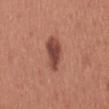Findings:
• body site: the abdomen
• size: ≈6 mm
• image: total-body-photography crop, ~15 mm field of view
• patient: male, approximately 45 years of age